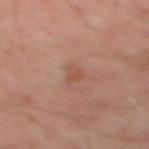Clinical impression:
Captured during whole-body skin photography for melanoma surveillance; the lesion was not biopsied.
Clinical summary:
A 15 mm close-up tile from a total-body photography series done for melanoma screening. On the mid back. The subject is a male aged 48–52.